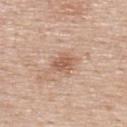The lesion was photographed on a routine skin check and not biopsied; there is no pathology result. The patient is a male approximately 55 years of age. The lesion-visualizer software estimated an area of roughly 4.5 mm², an outline eccentricity of about 0.65 (0 = round, 1 = elongated), and two-axis asymmetry of about 0.2. It also reported an automated nevus-likeness rating near 70 out of 100 and a detector confidence of about 100 out of 100 that the crop contains a lesion. Imaged with white-light lighting. Cropped from a total-body skin-imaging series; the visible field is about 15 mm. Located on the upper back. Longest diameter approximately 3 mm.